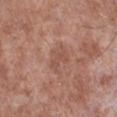Case summary:
• workup: total-body-photography surveillance lesion; no biopsy
• imaging modality: ~15 mm tile from a whole-body skin photo
• anatomic site: the right lower leg
• lesion size: ≈3.5 mm
• patient: male, aged approximately 55
• image-analysis metrics: a lesion area of about 6 mm², an outline eccentricity of about 0.7 (0 = round, 1 = elongated), and a symmetry-axis asymmetry near 0.35; a border-irregularity index near 4/10 and a color-variation rating of about 2/10; a nevus-likeness score of about 0/100 and a lesion-detection confidence of about 100/100
• illumination: white-light